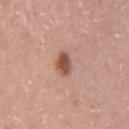Impression: This lesion was catalogued during total-body skin photography and was not selected for biopsy. Acquisition and patient details: Imaged with white-light lighting. Located on the right upper arm. A female subject, about 40 years old. A 15 mm close-up extracted from a 3D total-body photography capture. The recorded lesion diameter is about 2.5 mm. An algorithmic analysis of the crop reported a border-irregularity rating of about 1.5/10 and a within-lesion color-variation index near 3.5/10.